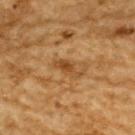{"biopsy_status": "not biopsied; imaged during a skin examination", "patient": {"sex": "male", "age_approx": "85+"}, "image": {"source": "total-body photography crop", "field_of_view_mm": 15}, "site": "back", "lighting": "cross-polarized", "automated_metrics": {"area_mm2_approx": 3.5, "eccentricity": 0.9, "shape_asymmetry": 0.35, "cielab_L": 40, "cielab_a": 19, "cielab_b": 36, "vs_skin_contrast_norm": 7.5, "border_irregularity_0_10": 4.0, "color_variation_0_10": 2.0, "peripheral_color_asymmetry": 0.5}}